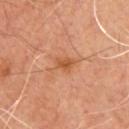Imaged during a routine full-body skin examination; the lesion was not biopsied and no histopathology is available.
The subject is a male about 55 years old.
The recorded lesion diameter is about 2.5 mm.
A 15 mm close-up extracted from a 3D total-body photography capture.
Automated tile analysis of the lesion measured an eccentricity of roughly 0.7 and a symmetry-axis asymmetry near 0.25.
The lesion is on the chest.